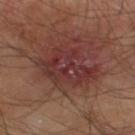Assessment:
Part of a total-body skin-imaging series; this lesion was reviewed on a skin check and was not flagged for biopsy.
Clinical summary:
The patient is a male in their mid- to late 60s. Approximately 6.5 mm at its widest. A close-up tile cropped from a whole-body skin photograph, about 15 mm across. The lesion is on the leg. The tile uses cross-polarized illumination.